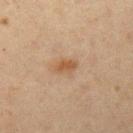The lesion was photographed on a routine skin check and not biopsied; there is no pathology result. An algorithmic analysis of the crop reported a border-irregularity index near 2.5/10, a within-lesion color-variation index near 1.5/10, and radial color variation of about 0.5. The tile uses cross-polarized illumination. A close-up tile cropped from a whole-body skin photograph, about 15 mm across. On the left upper arm. Approximately 2.5 mm at its widest. A female subject, roughly 40 years of age.